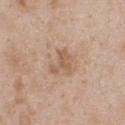Captured during whole-body skin photography for melanoma surveillance; the lesion was not biopsied.
Captured under white-light illumination.
A roughly 15 mm field-of-view crop from a total-body skin photograph.
A male patient, roughly 25 years of age.
Longest diameter approximately 3 mm.
On the upper back.
The total-body-photography lesion software estimated an average lesion color of about L≈58 a*≈18 b*≈32 (CIELAB), a lesion–skin lightness drop of about 8, and a lesion-to-skin contrast of about 6 (normalized; higher = more distinct). The analysis additionally found a border-irregularity index near 7/10 and a within-lesion color-variation index near 1.5/10. The analysis additionally found a detector confidence of about 100 out of 100 that the crop contains a lesion.